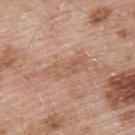  biopsy_status: not biopsied; imaged during a skin examination
  site: upper back
  patient:
    sex: male
    age_approx: 55
  image:
    source: total-body photography crop
    field_of_view_mm: 15
  lighting: white-light